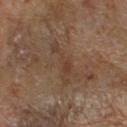The lesion was tiled from a total-body skin photograph and was not biopsied.
The lesion is on the right forearm.
A male subject approximately 65 years of age.
A region of skin cropped from a whole-body photographic capture, roughly 15 mm wide.